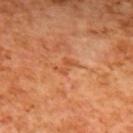| field | value |
|---|---|
| biopsy status | imaged on a skin check; not biopsied |
| subject | female, approximately 40 years of age |
| site | the upper back |
| image-analysis metrics | a lesion–skin lightness drop of about 7 and a lesion-to-skin contrast of about 5.5 (normalized; higher = more distinct); a border-irregularity index near 6/10, a color-variation rating of about 0/10, and radial color variation of about 0 |
| acquisition | ~15 mm tile from a whole-body skin photo |